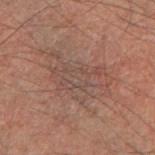  biopsy_status: not biopsied; imaged during a skin examination
  lesion_size:
    long_diameter_mm_approx: 8.0
  image:
    source: total-body photography crop
    field_of_view_mm: 15
  site: right forearm
  patient:
    sex: male
    age_approx: 60